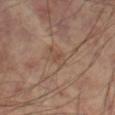Imaged during a routine full-body skin examination; the lesion was not biopsied and no histopathology is available.